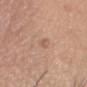| feature | finding |
|---|---|
| follow-up | imaged on a skin check; not biopsied |
| automated lesion analysis | a lesion area of about 1.5 mm², an eccentricity of roughly 0.8, and two-axis asymmetry of about 0.4; an average lesion color of about L≈58 a*≈20 b*≈30 (CIELAB), a lesion–skin lightness drop of about 7, and a normalized lesion–skin contrast near 5; a color-variation rating of about 0/10 and peripheral color asymmetry of about 0; a nevus-likeness score of about 0/100 |
| illumination | white-light |
| site | the head or neck |
| acquisition | total-body-photography crop, ~15 mm field of view |
| subject | male, roughly 60 years of age |
| lesion diameter | ≈2 mm |